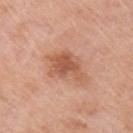{"biopsy_status": "not biopsied; imaged during a skin examination", "automated_metrics": {"vs_skin_darker_L": 10.0, "vs_skin_contrast_norm": 7.0, "border_irregularity_0_10": 4.0, "nevus_likeness_0_100": 35, "lesion_detection_confidence_0_100": 100}, "patient": {"sex": "male", "age_approx": 65}, "site": "right upper arm", "lesion_size": {"long_diameter_mm_approx": 5.5}, "image": {"source": "total-body photography crop", "field_of_view_mm": 15}, "lighting": "white-light"}